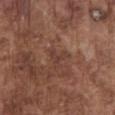Impression: The lesion was photographed on a routine skin check and not biopsied; there is no pathology result. Background: An algorithmic analysis of the crop reported a lesion area of about 3.5 mm², an outline eccentricity of about 0.3 (0 = round, 1 = elongated), and two-axis asymmetry of about 0.7. The analysis additionally found a border-irregularity rating of about 7.5/10, a color-variation rating of about 0/10, and a peripheral color-asymmetry measure near 0. And it measured an automated nevus-likeness rating near 0 out of 100. Imaged with white-light lighting. Located on the chest. Approximately 2.5 mm at its widest. A 15 mm close-up tile from a total-body photography series done for melanoma screening. A male patient aged 73 to 77.